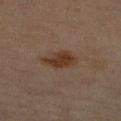Captured during whole-body skin photography for melanoma surveillance; the lesion was not biopsied. The lesion is located on the right upper arm. The tile uses cross-polarized illumination. A male patient, in their 70s. About 4 mm across. Cropped from a total-body skin-imaging series; the visible field is about 15 mm.